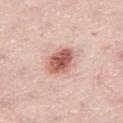<record>
<biopsy_status>not biopsied; imaged during a skin examination</biopsy_status>
<lesion_size>
  <long_diameter_mm_approx>4.0</long_diameter_mm_approx>
</lesion_size>
<site>right thigh</site>
<patient>
  <sex>male</sex>
  <age_approx>50</age_approx>
</patient>
<image>
  <source>total-body photography crop</source>
  <field_of_view_mm>15</field_of_view_mm>
</image>
<lighting>white-light</lighting>
</record>